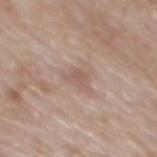| key | value |
|---|---|
| notes | total-body-photography surveillance lesion; no biopsy |
| patient | male, about 65 years old |
| illumination | white-light illumination |
| site | the mid back |
| image source | total-body-photography crop, ~15 mm field of view |
| lesion size | about 2.5 mm |
| TBP lesion metrics | a lesion area of about 3.5 mm² and an outline eccentricity of about 0.65 (0 = round, 1 = elongated); a border-irregularity index near 4/10, a within-lesion color-variation index near 2/10, and a peripheral color-asymmetry measure near 0.5 |